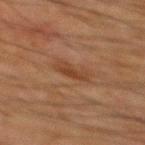| field | value |
|---|---|
| biopsy status | imaged on a skin check; not biopsied |
| lesion size | ~3 mm (longest diameter) |
| anatomic site | the mid back |
| patient | male, about 65 years old |
| image-analysis metrics | a shape eccentricity near 0.9 and two-axis asymmetry of about 0.3; a mean CIELAB color near L≈33 a*≈18 b*≈27, roughly 7 lightness units darker than nearby skin, and a lesion-to-skin contrast of about 7 (normalized; higher = more distinct); a detector confidence of about 100 out of 100 that the crop contains a lesion |
| imaging modality | total-body-photography crop, ~15 mm field of view |
| illumination | cross-polarized illumination |